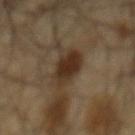{"biopsy_status": "not biopsied; imaged during a skin examination", "patient": {"sex": "male", "age_approx": 65}, "site": "back", "image": {"source": "total-body photography crop", "field_of_view_mm": 15}}